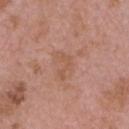{"biopsy_status": "not biopsied; imaged during a skin examination", "lesion_size": {"long_diameter_mm_approx": 3.5}, "patient": {"sex": "male", "age_approx": 75}, "lighting": "white-light", "image": {"source": "total-body photography crop", "field_of_view_mm": 15}, "site": "head or neck"}